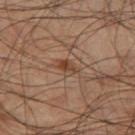Impression: This lesion was catalogued during total-body skin photography and was not selected for biopsy. Clinical summary: A close-up tile cropped from a whole-body skin photograph, about 15 mm across. This is a cross-polarized tile. A male subject aged 58–62. Automated image analysis of the tile measured a lesion–skin lightness drop of about 8 and a normalized lesion–skin contrast near 7.5. The software also gave a detector confidence of about 100 out of 100 that the crop contains a lesion. On the left thigh.